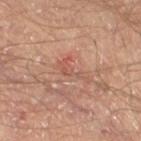Q: Was a biopsy performed?
A: no biopsy performed (imaged during a skin exam)
Q: What is the imaging modality?
A: 15 mm crop, total-body photography
Q: Where on the body is the lesion?
A: the right thigh
Q: Lesion size?
A: ≈4 mm
Q: Patient demographics?
A: male, aged approximately 65
Q: What lighting was used for the tile?
A: cross-polarized illumination